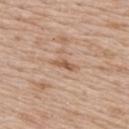Imaged during a routine full-body skin examination; the lesion was not biopsied and no histopathology is available.
The tile uses white-light illumination.
A 15 mm close-up extracted from a 3D total-body photography capture.
A female subject aged approximately 50.
Approximately 3 mm at its widest.
Located on the upper back.
The total-body-photography lesion software estimated a footprint of about 2.5 mm². The analysis additionally found a border-irregularity rating of about 4/10 and a color-variation rating of about 0/10.